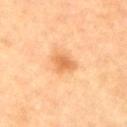Findings:
– follow-up — catalogued during a skin exam; not biopsied
– image source — ~15 mm tile from a whole-body skin photo
– illumination — cross-polarized illumination
– site — the arm
– patient — male, approximately 85 years of age or older
– size — ≈3 mm